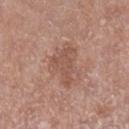<lesion>
<biopsy_status>not biopsied; imaged during a skin examination</biopsy_status>
<site>left lower leg</site>
<automated_metrics>
  <border_irregularity_0_10>6.5</border_irregularity_0_10>
</automated_metrics>
<image>
  <source>total-body photography crop</source>
  <field_of_view_mm>15</field_of_view_mm>
</image>
<lesion_size>
  <long_diameter_mm_approx>4.5</long_diameter_mm_approx>
</lesion_size>
<patient>
  <sex>female</sex>
  <age_approx>75</age_approx>
</patient>
<lighting>white-light</lighting>
</lesion>